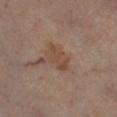This lesion was catalogued during total-body skin photography and was not selected for biopsy.
A male patient, aged 68–72.
Measured at roughly 4 mm in maximum diameter.
A 15 mm close-up tile from a total-body photography series done for melanoma screening.
Captured under cross-polarized illumination.
Located on the leg.